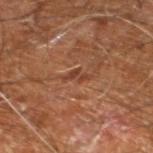biopsy status: catalogued during a skin exam; not biopsied | subject: male, roughly 60 years of age | acquisition: total-body-photography crop, ~15 mm field of view | lighting: cross-polarized | TBP lesion metrics: an eccentricity of roughly 0.9 and a symmetry-axis asymmetry near 0.55; roughly 7 lightness units darker than nearby skin and a normalized lesion–skin contrast near 6; lesion-presence confidence of about 60/100 | size: ≈2.5 mm | site: the right leg.